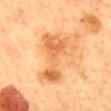Clinical impression: No biopsy was performed on this lesion — it was imaged during a full skin examination and was not determined to be concerning. Clinical summary: Measured at roughly 7 mm in maximum diameter. A female patient, aged 58 to 62. A 15 mm close-up extracted from a 3D total-body photography capture. From the mid back.